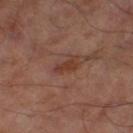biopsy_status: not biopsied; imaged during a skin examination
automated_metrics:
  color_variation_0_10: 0.5
  peripheral_color_asymmetry: 0.0
  nevus_likeness_0_100: 15
  lesion_detection_confidence_0_100: 100
site: left thigh
lesion_size:
  long_diameter_mm_approx: 3.0
patient:
  sex: male
  age_approx: 65
image:
  source: total-body photography crop
  field_of_view_mm: 15